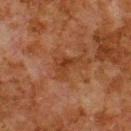{
  "biopsy_status": "not biopsied; imaged during a skin examination",
  "site": "upper back",
  "lesion_size": {
    "long_diameter_mm_approx": 4.0
  },
  "patient": {
    "sex": "male",
    "age_approx": 80
  },
  "lighting": "cross-polarized",
  "image": {
    "source": "total-body photography crop",
    "field_of_view_mm": 15
  }
}